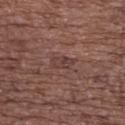Notes:
– workup · no biopsy performed (imaged during a skin exam)
– lesion diameter · ≈3 mm
– automated lesion analysis · a lesion area of about 3.5 mm², an outline eccentricity of about 0.9 (0 = round, 1 = elongated), and two-axis asymmetry of about 0.25; a lesion color around L≈39 a*≈19 b*≈21 in CIELAB, about 6 CIELAB-L* units darker than the surrounding skin, and a normalized lesion–skin contrast near 5.5
– lighting · white-light
– patient · female, roughly 75 years of age
– image source · ~15 mm crop, total-body skin-cancer survey
– site · the back